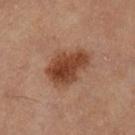Recorded during total-body skin imaging; not selected for excision or biopsy. The recorded lesion diameter is about 5 mm. This is a cross-polarized tile. On the right thigh. Cropped from a total-body skin-imaging series; the visible field is about 15 mm. A female subject aged approximately 60.